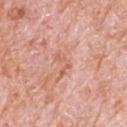The lesion was photographed on a routine skin check and not biopsied; there is no pathology result. Automated tile analysis of the lesion measured an area of roughly 3 mm², an eccentricity of roughly 0.85, and two-axis asymmetry of about 0.65. It also reported a mean CIELAB color near L≈62 a*≈25 b*≈31 and a lesion-to-skin contrast of about 5.5 (normalized; higher = more distinct). The software also gave a classifier nevus-likeness of about 0/100. From the upper back. The tile uses white-light illumination. A close-up tile cropped from a whole-body skin photograph, about 15 mm across. Measured at roughly 3 mm in maximum diameter. A male subject, approximately 80 years of age.